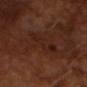Imaged during a routine full-body skin examination; the lesion was not biopsied and no histopathology is available.
A male subject, aged around 65.
About 5 mm across.
Imaged with cross-polarized lighting.
A close-up tile cropped from a whole-body skin photograph, about 15 mm across.